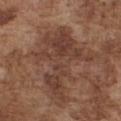Q: Was a biopsy performed?
A: no biopsy performed (imaged during a skin exam)
Q: What kind of image is this?
A: ~15 mm crop, total-body skin-cancer survey
Q: How large is the lesion?
A: ~8 mm (longest diameter)
Q: Automated lesion metrics?
A: an average lesion color of about L≈40 a*≈19 b*≈26 (CIELAB), about 8 CIELAB-L* units darker than the surrounding skin, and a normalized lesion–skin contrast near 7; a border-irregularity index near 9.5/10, internal color variation of about 4.5 on a 0–10 scale, and a peripheral color-asymmetry measure near 1.5
Q: What are the patient's age and sex?
A: male, aged 73 to 77
Q: Where on the body is the lesion?
A: the chest